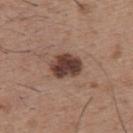- notes: total-body-photography surveillance lesion; no biopsy
- subject: male, aged 48 to 52
- illumination: white-light illumination
- image source: ~15 mm tile from a whole-body skin photo
- anatomic site: the upper back
- diameter: ≈4 mm